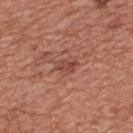No biopsy was performed on this lesion — it was imaged during a full skin examination and was not determined to be concerning.
A roughly 15 mm field-of-view crop from a total-body skin photograph.
A male subject, aged around 45.
The lesion is on the chest.